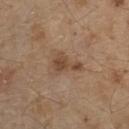Imaged during a routine full-body skin examination; the lesion was not biopsied and no histopathology is available. This is a white-light tile. Approximately 3.5 mm at its widest. The lesion is located on the front of the torso. A roughly 15 mm field-of-view crop from a total-body skin photograph. An algorithmic analysis of the crop reported a footprint of about 5.5 mm², an eccentricity of roughly 0.8, and two-axis asymmetry of about 0.55. The software also gave a classifier nevus-likeness of about 5/100. A male subject approximately 70 years of age.